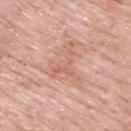{"image": {"source": "total-body photography crop", "field_of_view_mm": 15}, "patient": {"sex": "male", "age_approx": 55}, "site": "upper back"}